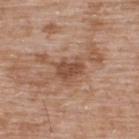Impression: The lesion was tiled from a total-body skin photograph and was not biopsied. Image and clinical context: A male patient, about 50 years old. Located on the upper back. This image is a 15 mm lesion crop taken from a total-body photograph.